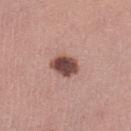<case>
  <biopsy_status>not biopsied; imaged during a skin examination</biopsy_status>
  <site>leg</site>
  <image>
    <source>total-body photography crop</source>
    <field_of_view_mm>15</field_of_view_mm>
  </image>
  <automated_metrics>
    <eccentricity>0.65</eccentricity>
    <shape_asymmetry>0.15</shape_asymmetry>
    <border_irregularity_0_10>1.5</border_irregularity_0_10>
    <color_variation_0_10>4.0</color_variation_0_10>
  </automated_metrics>
  <lesion_size>
    <long_diameter_mm_approx>3.5</long_diameter_mm_approx>
  </lesion_size>
  <patient>
    <sex>female</sex>
    <age_approx>35</age_approx>
  </patient>
</case>